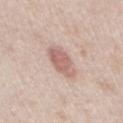notes: no biopsy performed (imaged during a skin exam) | acquisition: total-body-photography crop, ~15 mm field of view | illumination: white-light | body site: the chest | lesion diameter: about 4 mm | patient: male, approximately 40 years of age.